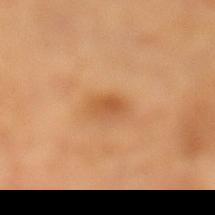notes: catalogued during a skin exam; not biopsied
location: the left lower leg
lesion size: ≈3 mm
image-analysis metrics: a lesion color around L≈57 a*≈25 b*≈43 in CIELAB, roughly 9 lightness units darker than nearby skin, and a normalized border contrast of about 6.5; a border-irregularity rating of about 1.5/10 and a peripheral color-asymmetry measure near 1; an automated nevus-likeness rating near 65 out of 100 and a lesion-detection confidence of about 100/100
patient: female, aged approximately 55
tile lighting: cross-polarized
image source: ~15 mm crop, total-body skin-cancer survey